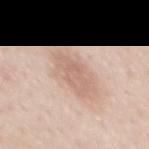biopsy_status: not biopsied; imaged during a skin examination
lighting: white-light
image:
  source: total-body photography crop
  field_of_view_mm: 15
site: mid back
lesion_size:
  long_diameter_mm_approx: 5.0
patient:
  sex: female
  age_approx: 40